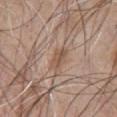Findings:
• follow-up: catalogued during a skin exam; not biopsied
• subject: male, aged 48 to 52
• illumination: white-light illumination
• acquisition: ~15 mm tile from a whole-body skin photo
• TBP lesion metrics: a footprint of about 4 mm², an outline eccentricity of about 0.75 (0 = round, 1 = elongated), and a shape-asymmetry score of about 0.25 (0 = symmetric); a border-irregularity index near 2.5/10, internal color variation of about 3.5 on a 0–10 scale, and peripheral color asymmetry of about 1.5
• size: ~3 mm (longest diameter)
• anatomic site: the chest